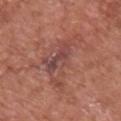  image:
    source: total-body photography crop
    field_of_view_mm: 15
  site: chest
  patient:
    sex: male
    age_approx: 75
  lesion_size:
    long_diameter_mm_approx: 7.5
  automated_metrics:
    area_mm2_approx: 16.0
    eccentricity: 0.9
    shape_asymmetry: 0.5
    cielab_L: 47
    cielab_a: 24
    cielab_b: 24
    vs_skin_darker_L: 7.0
    nevus_likeness_0_100: 0
    lesion_detection_confidence_0_100: 100